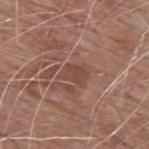Clinical impression:
Captured during whole-body skin photography for melanoma surveillance; the lesion was not biopsied.
Clinical summary:
Approximately 3 mm at its widest. This image is a 15 mm lesion crop taken from a total-body photograph. On the upper back. The subject is a male roughly 60 years of age. The total-body-photography lesion software estimated an eccentricity of roughly 0.65. The software also gave a lesion color around L≈45 a*≈20 b*≈26 in CIELAB, a lesion–skin lightness drop of about 7, and a lesion-to-skin contrast of about 5.5 (normalized; higher = more distinct). And it measured a within-lesion color-variation index near 1.5/10 and a peripheral color-asymmetry measure near 0.5. Imaged with white-light lighting.